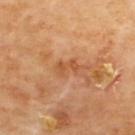| feature | finding |
|---|---|
| biopsy status | total-body-photography surveillance lesion; no biopsy |
| automated lesion analysis | lesion-presence confidence of about 100/100 |
| anatomic site | the upper back |
| lesion diameter | ≈2.5 mm |
| tile lighting | cross-polarized |
| imaging modality | ~15 mm crop, total-body skin-cancer survey |